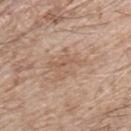Q: Was this lesion biopsied?
A: no biopsy performed (imaged during a skin exam)
Q: What is the anatomic site?
A: the front of the torso
Q: Patient demographics?
A: male, in their mid- to late 60s
Q: What kind of image is this?
A: 15 mm crop, total-body photography
Q: How large is the lesion?
A: ≈4 mm